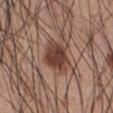Q: Was a biopsy performed?
A: imaged on a skin check; not biopsied
Q: What kind of image is this?
A: total-body-photography crop, ~15 mm field of view
Q: Where on the body is the lesion?
A: the abdomen
Q: Who is the patient?
A: male, about 55 years old